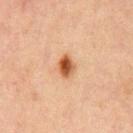biopsy status: total-body-photography surveillance lesion; no biopsy | site: the mid back | imaging modality: total-body-photography crop, ~15 mm field of view | patient: male, about 65 years old | illumination: cross-polarized | automated metrics: a footprint of about 5 mm².